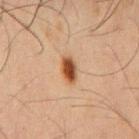Measured at roughly 3.5 mm in maximum diameter. A male patient, aged 58–62. A region of skin cropped from a whole-body photographic capture, roughly 15 mm wide. The lesion-visualizer software estimated a color-variation rating of about 5.5/10 and a peripheral color-asymmetry measure near 2.